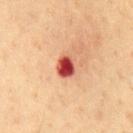Q: Was a biopsy performed?
A: imaged on a skin check; not biopsied
Q: How large is the lesion?
A: ~2.5 mm (longest diameter)
Q: Lesion location?
A: the chest
Q: How was the tile lit?
A: cross-polarized
Q: Patient demographics?
A: male, roughly 60 years of age
Q: Automated lesion metrics?
A: an average lesion color of about L≈45 a*≈34 b*≈30 (CIELAB), a lesion–skin lightness drop of about 19, and a normalized border contrast of about 13; an automated nevus-likeness rating near 0 out of 100 and a lesion-detection confidence of about 100/100
Q: What kind of image is this?
A: ~15 mm tile from a whole-body skin photo